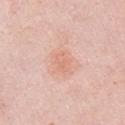No biopsy was performed on this lesion — it was imaged during a full skin examination and was not determined to be concerning.
An algorithmic analysis of the crop reported a footprint of about 4 mm², a shape eccentricity near 0.75, and two-axis asymmetry of about 0.25. The analysis additionally found a mean CIELAB color near L≈69 a*≈24 b*≈30 and roughly 6 lightness units darker than nearby skin. It also reported an automated nevus-likeness rating near 0 out of 100 and a detector confidence of about 100 out of 100 that the crop contains a lesion.
A 15 mm close-up tile from a total-body photography series done for melanoma screening.
This is a white-light tile.
On the right upper arm.
The lesion's longest dimension is about 2.5 mm.
A female subject aged approximately 65.